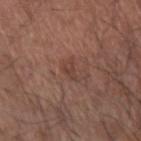Captured under white-light illumination. The lesion is located on the left forearm. The lesion-visualizer software estimated an eccentricity of roughly 0.9 and a symmetry-axis asymmetry near 0.45. It also reported border irregularity of about 4.5 on a 0–10 scale, internal color variation of about 1 on a 0–10 scale, and radial color variation of about 0. The software also gave a classifier nevus-likeness of about 0/100 and lesion-presence confidence of about 100/100. About 3 mm across. A male subject, aged 63 to 67. A region of skin cropped from a whole-body photographic capture, roughly 15 mm wide.